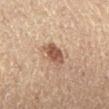Part of a total-body skin-imaging series; this lesion was reviewed on a skin check and was not flagged for biopsy. A region of skin cropped from a whole-body photographic capture, roughly 15 mm wide. This is a cross-polarized tile. Automated tile analysis of the lesion measured an outline eccentricity of about 0.55 (0 = round, 1 = elongated) and two-axis asymmetry of about 0.2. It also reported a color-variation rating of about 3/10. The software also gave a nevus-likeness score of about 85/100 and a lesion-detection confidence of about 100/100. From the leg. Measured at roughly 3 mm in maximum diameter. A male subject about 85 years old or older.